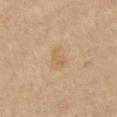Imaged during a routine full-body skin examination; the lesion was not biopsied and no histopathology is available. Automated tile analysis of the lesion measured an automated nevus-likeness rating near 0 out of 100. Measured at roughly 3.5 mm in maximum diameter. A 15 mm crop from a total-body photograph taken for skin-cancer surveillance. From the chest. A male subject, aged 43–47. This is a cross-polarized tile.